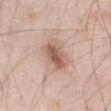notes: no biopsy performed (imaged during a skin exam)
location: the front of the torso
image: total-body-photography crop, ~15 mm field of view
patient: male, aged 68–72
diameter: about 4 mm